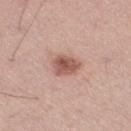Q: Was this lesion biopsied?
A: catalogued during a skin exam; not biopsied
Q: Patient demographics?
A: male, approximately 50 years of age
Q: Where on the body is the lesion?
A: the right thigh
Q: What did automated image analysis measure?
A: border irregularity of about 2 on a 0–10 scale and a within-lesion color-variation index near 3.5/10
Q: How was this image acquired?
A: total-body-photography crop, ~15 mm field of view
Q: What lighting was used for the tile?
A: white-light